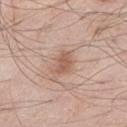Assessment: Part of a total-body skin-imaging series; this lesion was reviewed on a skin check and was not flagged for biopsy. Clinical summary: The tile uses white-light illumination. The lesion is located on the leg. The subject is a male aged 58–62. Cropped from a whole-body photographic skin survey; the tile spans about 15 mm.